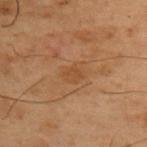<case>
  <biopsy_status>not biopsied; imaged during a skin examination</biopsy_status>
  <site>upper back</site>
  <patient>
    <sex>male</sex>
    <age_approx>55</age_approx>
  </patient>
  <lesion_size>
    <long_diameter_mm_approx>2.5</long_diameter_mm_approx>
  </lesion_size>
  <automated_metrics>
    <eccentricity>0.65</eccentricity>
    <border_irregularity_0_10>2.5</border_irregularity_0_10>
    <peripheral_color_asymmetry>1.0</peripheral_color_asymmetry>
    <nevus_likeness_0_100>0</nevus_likeness_0_100>
    <lesion_detection_confidence_0_100>100</lesion_detection_confidence_0_100>
  </automated_metrics>
  <lighting>cross-polarized</lighting>
  <image>
    <source>total-body photography crop</source>
    <field_of_view_mm>15</field_of_view_mm>
  </image>
</case>